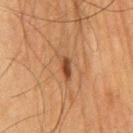Q: Is there a histopathology result?
A: no biopsy performed (imaged during a skin exam)
Q: Automated lesion metrics?
A: a mean CIELAB color near L≈39 a*≈20 b*≈30 and a lesion–skin lightness drop of about 10
Q: What is the anatomic site?
A: the chest
Q: How was the tile lit?
A: cross-polarized
Q: Who is the patient?
A: male, aged 63 to 67
Q: How was this image acquired?
A: 15 mm crop, total-body photography